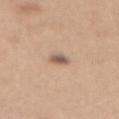Case summary:
* biopsy status: no biopsy performed (imaged during a skin exam)
* body site: the mid back
* patient: female, roughly 30 years of age
* acquisition: ~15 mm tile from a whole-body skin photo
* tile lighting: white-light
* lesion size: about 2.5 mm